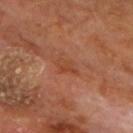No biopsy was performed on this lesion — it was imaged during a full skin examination and was not determined to be concerning. On the chest. The recorded lesion diameter is about 3 mm. The subject is a male aged approximately 60. A close-up tile cropped from a whole-body skin photograph, about 15 mm across. The tile uses cross-polarized illumination.